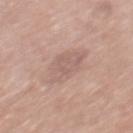| field | value |
|---|---|
| biopsy status | imaged on a skin check; not biopsied |
| image | 15 mm crop, total-body photography |
| subject | male, about 70 years old |
| anatomic site | the mid back |